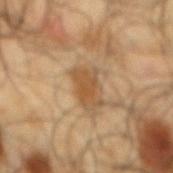This lesion was catalogued during total-body skin photography and was not selected for biopsy.
This is a cross-polarized tile.
Longest diameter approximately 3.5 mm.
A male subject about 65 years old.
A roughly 15 mm field-of-view crop from a total-body skin photograph.
The lesion is located on the mid back.